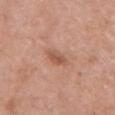Q: What are the patient's age and sex?
A: female, aged 58–62
Q: Where on the body is the lesion?
A: the chest
Q: Lesion size?
A: about 3 mm
Q: Illumination type?
A: white-light illumination
Q: How was this image acquired?
A: total-body-photography crop, ~15 mm field of view